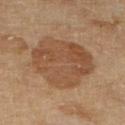Q: Was a biopsy performed?
A: catalogued during a skin exam; not biopsied
Q: Who is the patient?
A: female, about 55 years old
Q: What did automated image analysis measure?
A: a mean CIELAB color near L≈41 a*≈17 b*≈28, a lesion–skin lightness drop of about 8, and a normalized lesion–skin contrast near 6.5; a classifier nevus-likeness of about 10/100 and a lesion-detection confidence of about 100/100
Q: Where on the body is the lesion?
A: the leg
Q: What is the lesion's diameter?
A: ~7 mm (longest diameter)
Q: How was this image acquired?
A: 15 mm crop, total-body photography